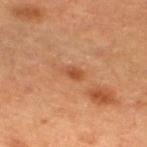Assessment:
Part of a total-body skin-imaging series; this lesion was reviewed on a skin check and was not flagged for biopsy.
Background:
A close-up tile cropped from a whole-body skin photograph, about 15 mm across. The lesion is located on the left lower leg. Approximately 2.5 mm at its widest. A female patient, about 60 years old.